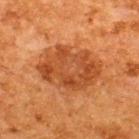Imaged during a routine full-body skin examination; the lesion was not biopsied and no histopathology is available.
Imaged with cross-polarized lighting.
A male patient aged 63–67.
Measured at roughly 7 mm in maximum diameter.
A 15 mm close-up tile from a total-body photography series done for melanoma screening.
The lesion is located on the upper back.